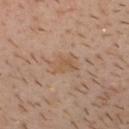Notes:
* acquisition: total-body-photography crop, ~15 mm field of view
* lesion size: ≈3.5 mm
* subject: male, roughly 30 years of age
* location: the head or neck